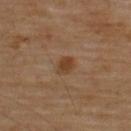Q: Was this lesion biopsied?
A: catalogued during a skin exam; not biopsied
Q: Patient demographics?
A: male, about 70 years old
Q: Illumination type?
A: cross-polarized illumination
Q: Automated lesion metrics?
A: an area of roughly 4 mm², an eccentricity of roughly 0.65, and two-axis asymmetry of about 0.2; internal color variation of about 2 on a 0–10 scale and radial color variation of about 0.5; a classifier nevus-likeness of about 85/100 and lesion-presence confidence of about 100/100
Q: What is the anatomic site?
A: the upper back
Q: How was this image acquired?
A: 15 mm crop, total-body photography
Q: How large is the lesion?
A: ~2.5 mm (longest diameter)